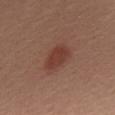{"biopsy_status": "not biopsied; imaged during a skin examination", "site": "upper back", "image": {"source": "total-body photography crop", "field_of_view_mm": 15}, "patient": {"sex": "male", "age_approx": 55}}